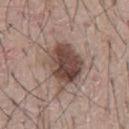Notes:
– notes — imaged on a skin check; not biopsied
– image source — total-body-photography crop, ~15 mm field of view
– site — the front of the torso
– subject — male, in their mid- to late 60s
– automated lesion analysis — a lesion area of about 18 mm², an eccentricity of roughly 0.6, and a symmetry-axis asymmetry near 0.25; a classifier nevus-likeness of about 85/100
– lesion size — ~5.5 mm (longest diameter)